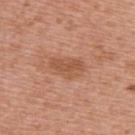biopsy status: catalogued during a skin exam; not biopsied | subject: female, about 40 years old | body site: the upper back | acquisition: total-body-photography crop, ~15 mm field of view | TBP lesion metrics: a lesion area of about 8 mm²; a border-irregularity index near 3/10, a within-lesion color-variation index near 2.5/10, and a peripheral color-asymmetry measure near 1 | lighting: white-light illumination.